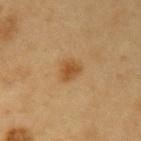{
  "biopsy_status": "not biopsied; imaged during a skin examination",
  "image": {
    "source": "total-body photography crop",
    "field_of_view_mm": 15
  },
  "patient": {
    "sex": "male",
    "age_approx": 60
  },
  "site": "left upper arm",
  "lighting": "cross-polarized"
}